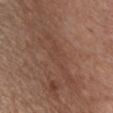{"biopsy_status": "not biopsied; imaged during a skin examination", "lighting": "white-light", "image": {"source": "total-body photography crop", "field_of_view_mm": 15}, "lesion_size": {"long_diameter_mm_approx": 8.0}, "site": "chest", "patient": {"sex": "male", "age_approx": 55}}